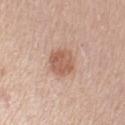The subject is a male about 60 years old.
From the left upper arm.
A region of skin cropped from a whole-body photographic capture, roughly 15 mm wide.